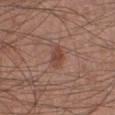Captured during whole-body skin photography for melanoma surveillance; the lesion was not biopsied. Cropped from a total-body skin-imaging series; the visible field is about 15 mm. Measured at roughly 3 mm in maximum diameter. A male subject aged 53 to 57. The lesion is on the right lower leg.